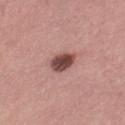The lesion was tiled from a total-body skin photograph and was not biopsied. The recorded lesion diameter is about 3 mm. This is a white-light tile. The subject is a female aged around 55. On the left thigh. A close-up tile cropped from a whole-body skin photograph, about 15 mm across.